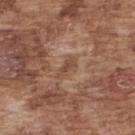body site: the back
size: ≈3 mm
subject: male, about 75 years old
imaging modality: ~15 mm crop, total-body skin-cancer survey
lighting: white-light illumination